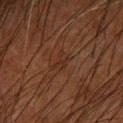notes: imaged on a skin check; not biopsied | site: the left forearm | tile lighting: cross-polarized illumination | lesion size: about 2.5 mm | image: ~15 mm crop, total-body skin-cancer survey | patient: male, aged around 65 | automated lesion analysis: a footprint of about 3.5 mm², an eccentricity of roughly 0.75, and a shape-asymmetry score of about 0.4 (0 = symmetric); a mean CIELAB color near L≈22 a*≈17 b*≈22, a lesion–skin lightness drop of about 4, and a lesion-to-skin contrast of about 4.5 (normalized; higher = more distinct); a nevus-likeness score of about 0/100 and a detector confidence of about 70 out of 100 that the crop contains a lesion.